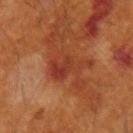Captured during whole-body skin photography for melanoma surveillance; the lesion was not biopsied. This is a cross-polarized tile. Approximately 5.5 mm at its widest. A region of skin cropped from a whole-body photographic capture, roughly 15 mm wide. From the right forearm. A male patient aged 58 to 62. The lesion-visualizer software estimated an outline eccentricity of about 0.85 (0 = round, 1 = elongated) and two-axis asymmetry of about 0.5. The analysis additionally found an average lesion color of about L≈36 a*≈28 b*≈32 (CIELAB) and roughly 7 lightness units darker than nearby skin. The software also gave a within-lesion color-variation index near 6/10 and peripheral color asymmetry of about 2. And it measured lesion-presence confidence of about 100/100.